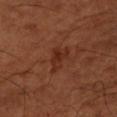Clinical impression:
This lesion was catalogued during total-body skin photography and was not selected for biopsy.
Clinical summary:
Cropped from a whole-body photographic skin survey; the tile spans about 15 mm. From the left forearm. About 3.5 mm across. The total-body-photography lesion software estimated a mean CIELAB color near L≈29 a*≈24 b*≈30, about 6 CIELAB-L* units darker than the surrounding skin, and a normalized border contrast of about 6.5. The analysis additionally found lesion-presence confidence of about 100/100. A male patient aged around 50. The tile uses cross-polarized illumination.